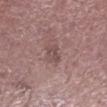lesion_size:
  long_diameter_mm_approx: 2.5
patient:
  sex: male
  age_approx: 65
site: left lower leg
automated_metrics:
  area_mm2_approx: 3.5
  eccentricity: 0.8
  border_irregularity_0_10: 3.0
  peripheral_color_asymmetry: 1.0
  nevus_likeness_0_100: 0
  lesion_detection_confidence_0_100: 100
lighting: white-light
image:
  source: total-body photography crop
  field_of_view_mm: 15